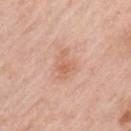A 15 mm crop from a total-body photograph taken for skin-cancer surveillance. About 4 mm across. This is a white-light tile. A female patient about 50 years old. The lesion is on the left upper arm.